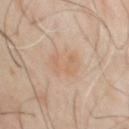Clinical impression:
Part of a total-body skin-imaging series; this lesion was reviewed on a skin check and was not flagged for biopsy.
Acquisition and patient details:
The lesion is on the chest. A roughly 15 mm field-of-view crop from a total-body skin photograph. A male subject, aged around 50.